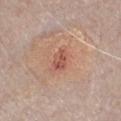<record>
<biopsy_status>not biopsied; imaged during a skin examination</biopsy_status>
<lesion_size>
  <long_diameter_mm_approx>2.5</long_diameter_mm_approx>
</lesion_size>
<automated_metrics>
  <eccentricity>0.8</eccentricity>
  <shape_asymmetry>0.2</shape_asymmetry>
  <cielab_L>54</cielab_L>
  <cielab_a>27</cielab_a>
  <cielab_b>29</cielab_b>
  <vs_skin_contrast_norm>6.5</vs_skin_contrast_norm>
  <color_variation_0_10>3.0</color_variation_0_10>
  <nevus_likeness_0_100>0</nevus_likeness_0_100>
  <lesion_detection_confidence_0_100>100</lesion_detection_confidence_0_100>
</automated_metrics>
<lighting>white-light</lighting>
<image>
  <source>total-body photography crop</source>
  <field_of_view_mm>15</field_of_view_mm>
</image>
<site>chest</site>
<patient>
  <sex>male</sex>
  <age_approx>70</age_approx>
</patient>
</record>